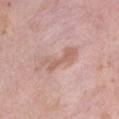  biopsy_status: not biopsied; imaged during a skin examination
  site: right thigh
  image:
    source: total-body photography crop
    field_of_view_mm: 15
  patient:
    sex: female
    age_approx: 40